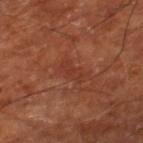Clinical impression:
Recorded during total-body skin imaging; not selected for excision or biopsy.
Clinical summary:
A subject about 65 years old. On the right lower leg. The tile uses cross-polarized illumination. A 15 mm crop from a total-body photograph taken for skin-cancer surveillance.